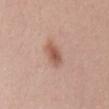Clinical impression: This lesion was catalogued during total-body skin photography and was not selected for biopsy. Context: The lesion is located on the chest. Cropped from a whole-body photographic skin survey; the tile spans about 15 mm. This is a white-light tile. Measured at roughly 3.5 mm in maximum diameter. The lesion-visualizer software estimated a lesion–skin lightness drop of about 11 and a normalized lesion–skin contrast near 7.5. And it measured a border-irregularity rating of about 2/10 and a color-variation rating of about 3.5/10. And it measured a nevus-likeness score of about 95/100 and a lesion-detection confidence of about 100/100. A female subject aged approximately 35.